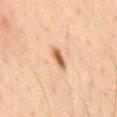biopsy_status: not biopsied; imaged during a skin examination
lighting: cross-polarized
site: lower back
patient:
  sex: male
  age_approx: 35
image:
  source: total-body photography crop
  field_of_view_mm: 15
automated_metrics:
  area_mm2_approx: 3.5
  shape_asymmetry: 0.2
  cielab_L: 57
  cielab_a: 22
  cielab_b: 34
  vs_skin_darker_L: 14.0
  color_variation_0_10: 3.0
  peripheral_color_asymmetry: 1.0